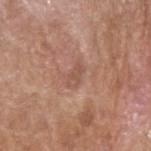Imaged with white-light lighting.
The patient is a male aged 63–67.
From the right upper arm.
A 15 mm close-up extracted from a 3D total-body photography capture.
The lesion's longest dimension is about 2.5 mm.